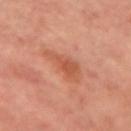Captured during whole-body skin photography for melanoma surveillance; the lesion was not biopsied.
The tile uses cross-polarized illumination.
Longest diameter approximately 5 mm.
Automated image analysis of the tile measured a color-variation rating of about 2.5/10 and a peripheral color-asymmetry measure near 0.5.
Cropped from a total-body skin-imaging series; the visible field is about 15 mm.
On the right upper arm.
A female patient approximately 60 years of age.